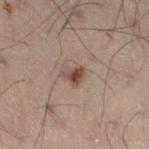Part of a total-body skin-imaging series; this lesion was reviewed on a skin check and was not flagged for biopsy. The lesion is located on the right thigh. Automated image analysis of the tile measured a border-irregularity rating of about 3/10, internal color variation of about 7 on a 0–10 scale, and peripheral color asymmetry of about 2.5. The software also gave a nevus-likeness score of about 95/100. A region of skin cropped from a whole-body photographic capture, roughly 15 mm wide. Imaged with cross-polarized lighting. A male patient roughly 50 years of age.